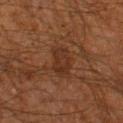Case summary:
- follow-up: imaged on a skin check; not biopsied
- image source: ~15 mm tile from a whole-body skin photo
- anatomic site: the leg
- TBP lesion metrics: a border-irregularity rating of about 2/10 and a within-lesion color-variation index near 2.5/10
- tile lighting: cross-polarized illumination
- subject: male, roughly 60 years of age